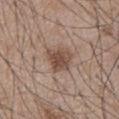workup — catalogued during a skin exam; not biopsied
image — ~15 mm tile from a whole-body skin photo
patient — male, about 55 years old
lighting — white-light
body site — the chest
lesion size — about 4 mm